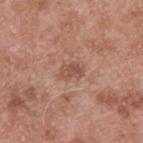Located on the back.
A 15 mm crop from a total-body photograph taken for skin-cancer surveillance.
The patient is a male aged around 55.